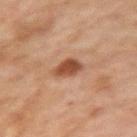Part of a total-body skin-imaging series; this lesion was reviewed on a skin check and was not flagged for biopsy.
A lesion tile, about 15 mm wide, cut from a 3D total-body photograph.
The subject is a female aged approximately 60.
Located on the left upper arm.